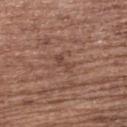| field | value |
|---|---|
| follow-up | catalogued during a skin exam; not biopsied |
| image | total-body-photography crop, ~15 mm field of view |
| site | the upper back |
| subject | female, aged around 65 |
| automated metrics | an automated nevus-likeness rating near 0 out of 100 and lesion-presence confidence of about 80/100 |
| illumination | white-light |
| lesion size | ~2.5 mm (longest diameter) |